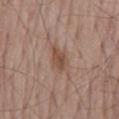A male patient about 65 years old.
Longest diameter approximately 2.5 mm.
A close-up tile cropped from a whole-body skin photograph, about 15 mm across.
This is a white-light tile.
An algorithmic analysis of the crop reported a border-irregularity rating of about 2/10, a color-variation rating of about 2.5/10, and radial color variation of about 1. The analysis additionally found a lesion-detection confidence of about 100/100.
The lesion is on the mid back.